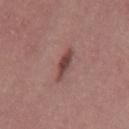follow-up = no biopsy performed (imaged during a skin exam); lesion size = about 3.5 mm; acquisition = 15 mm crop, total-body photography; anatomic site = the back; subject = male, aged approximately 40.